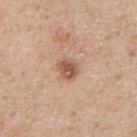  biopsy_status: not biopsied; imaged during a skin examination
  automated_metrics:
    cielab_L: 55
    cielab_a: 22
    cielab_b: 30
    vs_skin_darker_L: 12.0
    vs_skin_contrast_norm: 8.0
    nevus_likeness_0_100: 70
  site: right forearm
  patient:
    sex: female
    age_approx: 40
  lesion_size:
    long_diameter_mm_approx: 2.5
  image:
    source: total-body photography crop
    field_of_view_mm: 15
  lighting: white-light